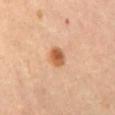notes=no biopsy performed (imaged during a skin exam).